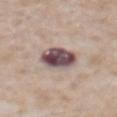notes: no biopsy performed (imaged during a skin exam); subject: male, aged approximately 65; lesion size: about 4 mm; body site: the abdomen; lighting: white-light illumination; acquisition: total-body-photography crop, ~15 mm field of view.